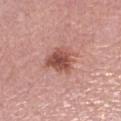Impression: Part of a total-body skin-imaging series; this lesion was reviewed on a skin check and was not flagged for biopsy. Background: The lesion-visualizer software estimated an average lesion color of about L≈52 a*≈25 b*≈26 (CIELAB), about 13 CIELAB-L* units darker than the surrounding skin, and a normalized lesion–skin contrast near 8.5. It also reported a detector confidence of about 100 out of 100 that the crop contains a lesion. On the right lower leg. A female patient roughly 70 years of age. Cropped from a total-body skin-imaging series; the visible field is about 15 mm. The tile uses white-light illumination. Approximately 4 mm at its widest.